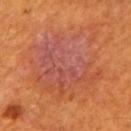- workup · catalogued during a skin exam; not biopsied
- lighting · cross-polarized illumination
- imaging modality · total-body-photography crop, ~15 mm field of view
- anatomic site · the back
- diameter · about 9 mm
- patient · male, approximately 60 years of age